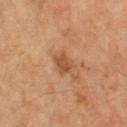{
  "biopsy_status": "not biopsied; imaged during a skin examination",
  "lesion_size": {
    "long_diameter_mm_approx": 2.5
  },
  "automated_metrics": {
    "area_mm2_approx": 4.0,
    "shape_asymmetry": 0.3
  },
  "patient": {
    "sex": "female",
    "age_approx": 55
  },
  "site": "left thigh",
  "lighting": "cross-polarized",
  "image": {
    "source": "total-body photography crop",
    "field_of_view_mm": 15
  }
}